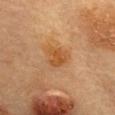Clinical impression:
Part of a total-body skin-imaging series; this lesion was reviewed on a skin check and was not flagged for biopsy.
Background:
A female subject, aged approximately 60. Captured under cross-polarized illumination. Located on the mid back. Longest diameter approximately 3 mm. Cropped from a whole-body photographic skin survey; the tile spans about 15 mm.